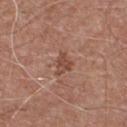| field | value |
|---|---|
| biopsy status | catalogued during a skin exam; not biopsied |
| image-analysis metrics | a lesion color around L≈47 a*≈22 b*≈28 in CIELAB, about 9 CIELAB-L* units darker than the surrounding skin, and a normalized border contrast of about 7 |
| subject | male, approximately 55 years of age |
| body site | the chest |
| acquisition | ~15 mm tile from a whole-body skin photo |